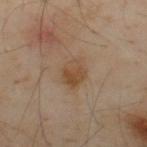notes: total-body-photography surveillance lesion; no biopsy | subject: male, roughly 55 years of age | site: the upper back | TBP lesion metrics: a mean CIELAB color near L≈48 a*≈16 b*≈31 and a normalized lesion–skin contrast near 7; a nevus-likeness score of about 40/100 and a lesion-detection confidence of about 100/100 | acquisition: ~15 mm crop, total-body skin-cancer survey | size: ≈3.5 mm.